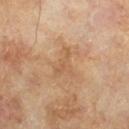Assessment: Recorded during total-body skin imaging; not selected for excision or biopsy. Clinical summary: The lesion-visualizer software estimated an area of roughly 4.5 mm², a shape eccentricity near 0.75, and a symmetry-axis asymmetry near 0.7. The software also gave a lesion color around L≈58 a*≈20 b*≈35 in CIELAB and a normalized border contrast of about 4.5. It also reported a border-irregularity index near 7.5/10, a color-variation rating of about 0.5/10, and peripheral color asymmetry of about 0. And it measured a classifier nevus-likeness of about 0/100 and lesion-presence confidence of about 100/100. A male subject, aged 63 to 67. A 15 mm close-up extracted from a 3D total-body photography capture. From the leg. About 3.5 mm across. The tile uses cross-polarized illumination.